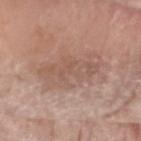Imaged during a routine full-body skin examination; the lesion was not biopsied and no histopathology is available.